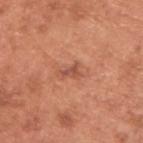From the upper back.
The subject is a male aged approximately 65.
A close-up tile cropped from a whole-body skin photograph, about 15 mm across.
An algorithmic analysis of the crop reported a lesion color around L≈53 a*≈27 b*≈32 in CIELAB, roughly 9 lightness units darker than nearby skin, and a normalized lesion–skin contrast near 6.5. The software also gave a border-irregularity index near 5/10, a within-lesion color-variation index near 0.5/10, and peripheral color asymmetry of about 0.